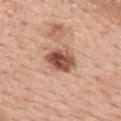– follow-up — total-body-photography surveillance lesion; no biopsy
– size — ~4 mm (longest diameter)
– anatomic site — the upper back
– image — ~15 mm crop, total-body skin-cancer survey
– automated metrics — a lesion color around L≈52 a*≈23 b*≈30 in CIELAB; a detector confidence of about 100 out of 100 that the crop contains a lesion
– patient — female, aged approximately 60